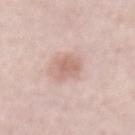Imaged during a routine full-body skin examination; the lesion was not biopsied and no histopathology is available. The recorded lesion diameter is about 3.5 mm. The lesion is located on the abdomen. The lesion-visualizer software estimated a lesion area of about 6.5 mm², an outline eccentricity of about 0.7 (0 = round, 1 = elongated), and a symmetry-axis asymmetry near 0.3. The analysis additionally found a mean CIELAB color near L≈65 a*≈20 b*≈25 and a lesion–skin lightness drop of about 10. And it measured border irregularity of about 3 on a 0–10 scale, internal color variation of about 2.5 on a 0–10 scale, and peripheral color asymmetry of about 1. The tile uses white-light illumination. Cropped from a total-body skin-imaging series; the visible field is about 15 mm. A male subject, about 60 years old.